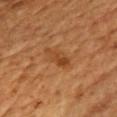Assessment: Imaged during a routine full-body skin examination; the lesion was not biopsied and no histopathology is available. Clinical summary: Captured under cross-polarized illumination. The recorded lesion diameter is about 3.5 mm. A male subject, in their 60s. Cropped from a whole-body photographic skin survey; the tile spans about 15 mm. The lesion is on the chest.